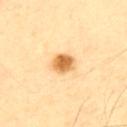Impression: Recorded during total-body skin imaging; not selected for excision or biopsy. Acquisition and patient details: The lesion-visualizer software estimated an average lesion color of about L≈69 a*≈22 b*≈48 (CIELAB), a lesion–skin lightness drop of about 16, and a normalized lesion–skin contrast near 10. And it measured a border-irregularity index near 1/10 and peripheral color asymmetry of about 1. A region of skin cropped from a whole-body photographic capture, roughly 15 mm wide. From the upper back. The patient is a male roughly 65 years of age. The lesion's longest dimension is about 2.5 mm.